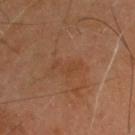Assessment:
Recorded during total-body skin imaging; not selected for excision or biopsy.
Background:
The lesion-visualizer software estimated a lesion area of about 4.5 mm², an eccentricity of roughly 0.9, and two-axis asymmetry of about 0.6. And it measured a mean CIELAB color near L≈39 a*≈20 b*≈31 and a normalized border contrast of about 4.5. And it measured a nevus-likeness score of about 0/100. The patient is a male in their 60s. A 15 mm close-up tile from a total-body photography series done for melanoma screening. From the head or neck.